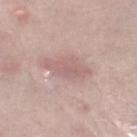No biopsy was performed on this lesion — it was imaged during a full skin examination and was not determined to be concerning. A 15 mm close-up extracted from a 3D total-body photography capture. The lesion is located on the right thigh. This is a white-light tile. The lesion's longest dimension is about 5.5 mm. The lesion-visualizer software estimated a footprint of about 9 mm² and an outline eccentricity of about 0.9 (0 = round, 1 = elongated). The analysis additionally found an average lesion color of about L≈61 a*≈19 b*≈21 (CIELAB), a lesion–skin lightness drop of about 8, and a lesion-to-skin contrast of about 5.5 (normalized; higher = more distinct). A male patient aged 63–67.